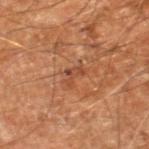{
  "biopsy_status": "not biopsied; imaged during a skin examination",
  "lesion_size": {
    "long_diameter_mm_approx": 2.5
  },
  "lighting": "cross-polarized",
  "image": {
    "source": "total-body photography crop",
    "field_of_view_mm": 15
  },
  "site": "leg",
  "automated_metrics": {
    "vs_skin_darker_L": 8.0,
    "border_irregularity_0_10": 7.0,
    "peripheral_color_asymmetry": 0.0,
    "nevus_likeness_0_100": 0,
    "lesion_detection_confidence_0_100": 100
  },
  "patient": {
    "sex": "male",
    "age_approx": 60
  }
}